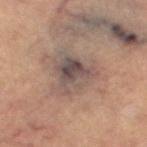Q: Was a biopsy performed?
A: imaged on a skin check; not biopsied
Q: How was the tile lit?
A: cross-polarized
Q: Who is the patient?
A: female, aged approximately 65
Q: How was this image acquired?
A: ~15 mm tile from a whole-body skin photo
Q: Where on the body is the lesion?
A: the left thigh
Q: What did automated image analysis measure?
A: an area of roughly 12 mm², an outline eccentricity of about 0.45 (0 = round, 1 = elongated), and a symmetry-axis asymmetry near 0.4; a border-irregularity index near 4.5/10 and peripheral color asymmetry of about 2
Q: How large is the lesion?
A: ≈4.5 mm